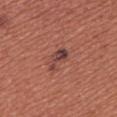biopsy status: total-body-photography surveillance lesion; no biopsy | anatomic site: the right upper arm | TBP lesion metrics: an eccentricity of roughly 0.85 and a shape-asymmetry score of about 0.45 (0 = symmetric); a lesion color around L≈42 a*≈24 b*≈23 in CIELAB, roughly 10 lightness units darker than nearby skin, and a normalized border contrast of about 9; a border-irregularity index near 5/10, a color-variation rating of about 6/10, and radial color variation of about 2; an automated nevus-likeness rating near 20 out of 100 and a lesion-detection confidence of about 100/100 | subject: female, aged around 35 | lesion size: ≈3.5 mm | imaging modality: ~15 mm tile from a whole-body skin photo.